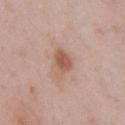Assessment: The lesion was photographed on a routine skin check and not biopsied; there is no pathology result. Image and clinical context: The subject is a male roughly 55 years of age. The lesion is on the chest. Cropped from a total-body skin-imaging series; the visible field is about 15 mm.